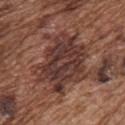Impression:
Captured during whole-body skin photography for melanoma surveillance; the lesion was not biopsied.
Background:
Cropped from a whole-body photographic skin survey; the tile spans about 15 mm. A male subject, roughly 75 years of age. From the chest. This is a white-light tile.